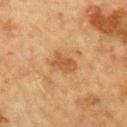Impression: Recorded during total-body skin imaging; not selected for excision or biopsy. Context: Cropped from a whole-body photographic skin survey; the tile spans about 15 mm. From the mid back. A male subject roughly 70 years of age. About 3 mm across.